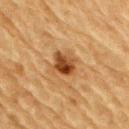Captured during whole-body skin photography for melanoma surveillance; the lesion was not biopsied.